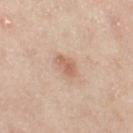| feature | finding |
|---|---|
| automated metrics | an eccentricity of roughly 0.75 and a shape-asymmetry score of about 0.25 (0 = symmetric); a mean CIELAB color near L≈62 a*≈21 b*≈30, roughly 9 lightness units darker than nearby skin, and a lesion-to-skin contrast of about 6 (normalized; higher = more distinct); a border-irregularity rating of about 2/10, internal color variation of about 3 on a 0–10 scale, and a peripheral color-asymmetry measure near 1; lesion-presence confidence of about 100/100 |
| lighting | cross-polarized |
| location | the left thigh |
| image source | ~15 mm crop, total-body skin-cancer survey |
| subject | female, aged approximately 55 |
| diameter | ~3 mm (longest diameter) |